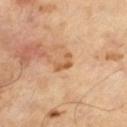notes: no biopsy performed (imaged during a skin exam) | acquisition: ~15 mm tile from a whole-body skin photo | subject: male, aged approximately 65 | size: about 2.5 mm.